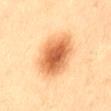follow-up — total-body-photography surveillance lesion; no biopsy
lighting — cross-polarized
site — the lower back
patient — male, in their mid-50s
image — ~15 mm tile from a whole-body skin photo